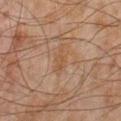Notes:
* workup: catalogued during a skin exam; not biopsied
* anatomic site: the left lower leg
* image: total-body-photography crop, ~15 mm field of view
* lesion size: ~3.5 mm (longest diameter)
* patient: male, aged around 45